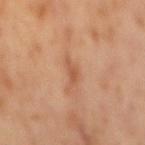Findings:
* workup: catalogued during a skin exam; not biopsied
* acquisition: 15 mm crop, total-body photography
* patient: male, aged 63 to 67